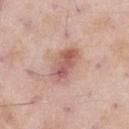Part of a total-body skin-imaging series; this lesion was reviewed on a skin check and was not flagged for biopsy.
The patient is a male approximately 55 years of age.
The recorded lesion diameter is about 5 mm.
This image is a 15 mm lesion crop taken from a total-body photograph.
The lesion is on the left thigh.
The lesion-visualizer software estimated a footprint of about 10 mm² and a shape eccentricity near 0.85. The analysis additionally found a lesion–skin lightness drop of about 12 and a normalized border contrast of about 7.5. It also reported border irregularity of about 2.5 on a 0–10 scale, internal color variation of about 8.5 on a 0–10 scale, and a peripheral color-asymmetry measure near 3.5.